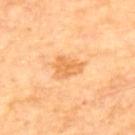Assessment:
The lesion was tiled from a total-body skin photograph and was not biopsied.
Clinical summary:
A male subject about 70 years old. An algorithmic analysis of the crop reported a lesion–skin lightness drop of about 9 and a normalized lesion–skin contrast near 6.5. Cropped from a whole-body photographic skin survey; the tile spans about 15 mm. Located on the upper back.